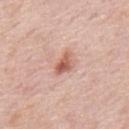workup=no biopsy performed (imaged during a skin exam) | size=≈3 mm | location=the mid back | subject=male, aged 73 to 77 | acquisition=total-body-photography crop, ~15 mm field of view.